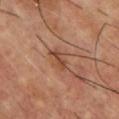Impression: The lesion was photographed on a routine skin check and not biopsied; there is no pathology result. Acquisition and patient details: Imaged with cross-polarized lighting. The patient is a male aged approximately 55. About 2.5 mm across. From the front of the torso. Automated image analysis of the tile measured an automated nevus-likeness rating near 5 out of 100 and a lesion-detection confidence of about 100/100. A 15 mm close-up tile from a total-body photography series done for melanoma screening.